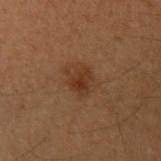site: the left upper arm
subject: female, about 50 years old
imaging modality: ~15 mm tile from a whole-body skin photo